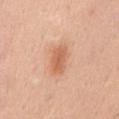biopsy_status: not biopsied; imaged during a skin examination
patient:
  sex: female
  age_approx: 55
lesion_size:
  long_diameter_mm_approx: 4.0
image:
  source: total-body photography crop
  field_of_view_mm: 15
site: abdomen
lighting: white-light